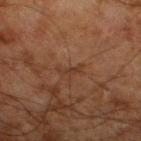This lesion was catalogued during total-body skin photography and was not selected for biopsy.
A roughly 15 mm field-of-view crop from a total-body skin photograph.
Located on the right thigh.
Automated tile analysis of the lesion measured a lesion area of about 2.5 mm², a shape eccentricity near 0.9, and a symmetry-axis asymmetry near 0.3. And it measured about 4 CIELAB-L* units darker than the surrounding skin and a normalized border contrast of about 5. The analysis additionally found a border-irregularity index near 4/10, internal color variation of about 0 on a 0–10 scale, and peripheral color asymmetry of about 0. The software also gave a nevus-likeness score of about 0/100.
A male subject approximately 80 years of age.
Imaged with cross-polarized lighting.
The recorded lesion diameter is about 2.5 mm.